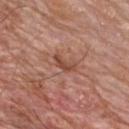<record>
<biopsy_status>not biopsied; imaged during a skin examination</biopsy_status>
<image>
  <source>total-body photography crop</source>
  <field_of_view_mm>15</field_of_view_mm>
</image>
<patient>
  <sex>male</sex>
  <age_approx>60</age_approx>
</patient>
<lesion_size>
  <long_diameter_mm_approx>3.0</long_diameter_mm_approx>
</lesion_size>
<lighting>white-light</lighting>
<site>chest</site>
</record>